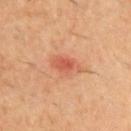Findings:
• patient: male, aged approximately 60
• image: ~15 mm tile from a whole-body skin photo
• illumination: cross-polarized illumination
• location: the chest
• diameter: ~4 mm (longest diameter)